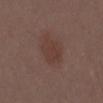<lesion>
  <biopsy_status>not biopsied; imaged during a skin examination</biopsy_status>
  <lighting>white-light</lighting>
  <image>
    <source>total-body photography crop</source>
    <field_of_view_mm>15</field_of_view_mm>
  </image>
  <lesion_size>
    <long_diameter_mm_approx>3.5</long_diameter_mm_approx>
  </lesion_size>
  <patient>
    <sex>female</sex>
    <age_approx>30</age_approx>
  </patient>
  <site>mid back</site>
</lesion>